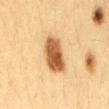Notes:
- biopsy status · no biopsy performed (imaged during a skin exam)
- anatomic site · the mid back
- patient · female, aged 28–32
- lighting · cross-polarized illumination
- image source · ~15 mm crop, total-body skin-cancer survey